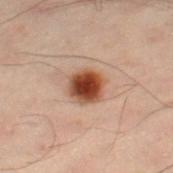workup: imaged on a skin check; not biopsied
acquisition: total-body-photography crop, ~15 mm field of view
lighting: cross-polarized
subject: male, aged around 50
anatomic site: the left leg
automated lesion analysis: two-axis asymmetry of about 0.15; a border-irregularity rating of about 1.5/10, a within-lesion color-variation index near 6/10, and a peripheral color-asymmetry measure near 1.5
lesion size: ~3.5 mm (longest diameter)